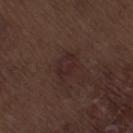Notes:
- biopsy status: catalogued during a skin exam; not biopsied
- lesion diameter: about 3.5 mm
- site: the right thigh
- imaging modality: ~15 mm crop, total-body skin-cancer survey
- lighting: white-light
- patient: male, in their 70s